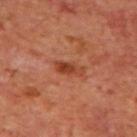Impression: Part of a total-body skin-imaging series; this lesion was reviewed on a skin check and was not flagged for biopsy. Image and clinical context: Cropped from a total-body skin-imaging series; the visible field is about 15 mm. On the mid back. The lesion-visualizer software estimated a lesion color around L≈41 a*≈29 b*≈34 in CIELAB, a lesion–skin lightness drop of about 10, and a normalized lesion–skin contrast near 8. And it measured border irregularity of about 3.5 on a 0–10 scale and internal color variation of about 2.5 on a 0–10 scale. It also reported an automated nevus-likeness rating near 75 out of 100 and a detector confidence of about 100 out of 100 that the crop contains a lesion. A male patient roughly 70 years of age. This is a cross-polarized tile.